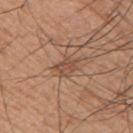follow-up = catalogued during a skin exam; not biopsied
imaging modality = ~15 mm tile from a whole-body skin photo
illumination = white-light illumination
patient = male, aged 53 to 57
automated lesion analysis = a footprint of about 4.5 mm² and a symmetry-axis asymmetry near 0.35
anatomic site = the right upper arm